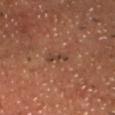Captured during whole-body skin photography for melanoma surveillance; the lesion was not biopsied. The lesion is on the head or neck. A male patient, aged 58 to 62. A roughly 15 mm field-of-view crop from a total-body skin photograph.